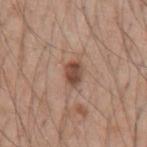Recorded during total-body skin imaging; not selected for excision or biopsy. The lesion's longest dimension is about 3 mm. On the left upper arm. Imaged with white-light lighting. A male subject, in their 50s. The lesion-visualizer software estimated a lesion area of about 5 mm², an outline eccentricity of about 0.75 (0 = round, 1 = elongated), and a shape-asymmetry score of about 0.2 (0 = symmetric). The analysis additionally found a border-irregularity index near 2/10, a color-variation rating of about 3/10, and a peripheral color-asymmetry measure near 1. A 15 mm crop from a total-body photograph taken for skin-cancer surveillance.